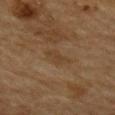notes — catalogued during a skin exam; not biopsied | illumination — cross-polarized | anatomic site — the back | imaging modality — 15 mm crop, total-body photography | subject — male, aged around 85 or older.